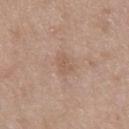The lesion is on the chest. About 2.5 mm across. Captured under white-light illumination. The lesion-visualizer software estimated a lesion area of about 3.5 mm², a shape eccentricity near 0.85, and a shape-asymmetry score of about 0.3 (0 = symmetric). And it measured a mean CIELAB color near L≈57 a*≈17 b*≈28, a lesion–skin lightness drop of about 6, and a lesion-to-skin contrast of about 5 (normalized; higher = more distinct). The software also gave an automated nevus-likeness rating near 0 out of 100 and a detector confidence of about 100 out of 100 that the crop contains a lesion. Cropped from a total-body skin-imaging series; the visible field is about 15 mm. The patient is a male aged around 50.